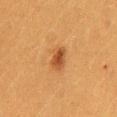Findings:
- notes · imaged on a skin check; not biopsied
- anatomic site · the mid back
- image-analysis metrics · a lesion area of about 4.5 mm² and two-axis asymmetry of about 0.2; about 10 CIELAB-L* units darker than the surrounding skin and a normalized lesion–skin contrast near 8.5; a border-irregularity index near 2/10 and a within-lesion color-variation index near 3.5/10; an automated nevus-likeness rating near 95 out of 100 and a detector confidence of about 100 out of 100 that the crop contains a lesion
- diameter · about 3 mm
- subject · female, about 40 years old
- tile lighting · cross-polarized
- imaging modality · ~15 mm tile from a whole-body skin photo